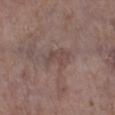| feature | finding |
|---|---|
| follow-up | imaged on a skin check; not biopsied |
| lesion diameter | about 3.5 mm |
| automated metrics | an area of roughly 6.5 mm², a shape eccentricity near 0.8, and two-axis asymmetry of about 0.35; an automated nevus-likeness rating near 0 out of 100 and a lesion-detection confidence of about 70/100 |
| patient | female, aged 83 or older |
| tile lighting | white-light illumination |
| acquisition | total-body-photography crop, ~15 mm field of view |
| body site | the left lower leg |